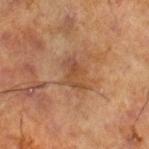follow-up: total-body-photography surveillance lesion; no biopsy
image-analysis metrics: a lesion area of about 5 mm²; border irregularity of about 6.5 on a 0–10 scale and peripheral color asymmetry of about 0.5
acquisition: ~15 mm tile from a whole-body skin photo
lesion diameter: about 3.5 mm
subject: male, in their 70s
location: the right lower leg
illumination: cross-polarized illumination